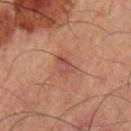site = the left upper arm | image source = ~15 mm crop, total-body skin-cancer survey | subject = male, approximately 50 years of age | size = ≈3 mm.